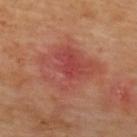follow-up: total-body-photography surveillance lesion; no biopsy | acquisition: ~15 mm crop, total-body skin-cancer survey | location: the upper back | tile lighting: cross-polarized illumination.